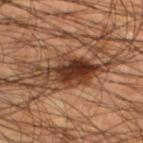Q: Was a biopsy performed?
A: no biopsy performed (imaged during a skin exam)
Q: Patient demographics?
A: male, aged around 45
Q: How was this image acquired?
A: 15 mm crop, total-body photography
Q: Where on the body is the lesion?
A: the left lower leg